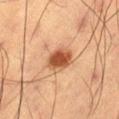Q: Was a biopsy performed?
A: catalogued during a skin exam; not biopsied
Q: What kind of image is this?
A: 15 mm crop, total-body photography
Q: Patient demographics?
A: male, aged approximately 65
Q: What lighting was used for the tile?
A: cross-polarized illumination
Q: What is the anatomic site?
A: the right thigh
Q: Lesion size?
A: ≈3 mm